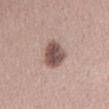| feature | finding |
|---|---|
| follow-up | imaged on a skin check; not biopsied |
| image | ~15 mm crop, total-body skin-cancer survey |
| diameter | ≈4 mm |
| site | the abdomen |
| subject | female, aged 43–47 |
| illumination | white-light illumination |